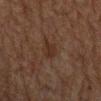Located on the mid back. The total-body-photography lesion software estimated a detector confidence of about 90 out of 100 that the crop contains a lesion. This is a cross-polarized tile. A male subject aged around 80. Longest diameter approximately 3 mm. A region of skin cropped from a whole-body photographic capture, roughly 15 mm wide.